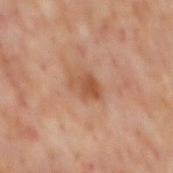A region of skin cropped from a whole-body photographic capture, roughly 15 mm wide. A male patient, in their mid- to late 60s. An algorithmic analysis of the crop reported an area of roughly 5 mm² and two-axis asymmetry of about 0.3. The lesion is located on the mid back.